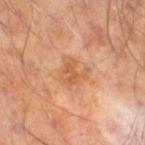notes = total-body-photography surveillance lesion; no biopsy | image = 15 mm crop, total-body photography | illumination = cross-polarized | diameter = ≈3.5 mm | subject = male, roughly 55 years of age | image-analysis metrics = a lesion color around L≈55 a*≈23 b*≈36 in CIELAB, about 7 CIELAB-L* units darker than the surrounding skin, and a normalized lesion–skin contrast near 5.5; lesion-presence confidence of about 100/100 | anatomic site = the right thigh.